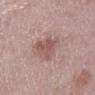Captured during whole-body skin photography for melanoma surveillance; the lesion was not biopsied. About 5 mm across. Captured under white-light illumination. A close-up tile cropped from a whole-body skin photograph, about 15 mm across. A male patient, approximately 70 years of age. Located on the mid back.